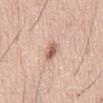Findings:
- location · the lower back
- acquisition · ~15 mm crop, total-body skin-cancer survey
- subject · male, aged approximately 60
- diameter · ~2.5 mm (longest diameter)